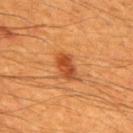Captured during whole-body skin photography for melanoma surveillance; the lesion was not biopsied. The patient is a male about 60 years old. About 3.5 mm across. A region of skin cropped from a whole-body photographic capture, roughly 15 mm wide. From the mid back. Captured under cross-polarized illumination.